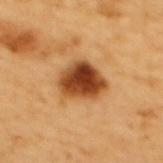biopsy status = imaged on a skin check; not biopsied
imaging modality = ~15 mm tile from a whole-body skin photo
subject = female, aged approximately 55
automated metrics = a classifier nevus-likeness of about 100/100 and a detector confidence of about 100 out of 100 that the crop contains a lesion
size = about 5 mm
body site = the upper back
lighting = cross-polarized